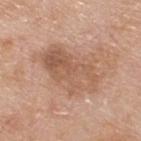biopsy_status: not biopsied; imaged during a skin examination
image:
  source: total-body photography crop
  field_of_view_mm: 15
site: upper back
patient:
  sex: male
  age_approx: 80